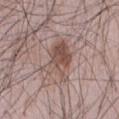A roughly 15 mm field-of-view crop from a total-body skin photograph. From the abdomen. An algorithmic analysis of the crop reported a border-irregularity rating of about 5.5/10, internal color variation of about 6 on a 0–10 scale, and a peripheral color-asymmetry measure near 2. The analysis additionally found a classifier nevus-likeness of about 40/100. Approximately 5.5 mm at its widest. This is a white-light tile. A male patient, aged 48–52.